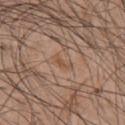* follow-up — imaged on a skin check; not biopsied
* acquisition — 15 mm crop, total-body photography
* patient — male, aged approximately 45
* diameter — about 2.5 mm
* site — the chest